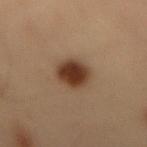Impression: Recorded during total-body skin imaging; not selected for excision or biopsy. Clinical summary: Measured at roughly 4.5 mm in maximum diameter. From the lower back. A lesion tile, about 15 mm wide, cut from a 3D total-body photograph. The lesion-visualizer software estimated an area of roughly 10 mm², an outline eccentricity of about 0.75 (0 = round, 1 = elongated), and two-axis asymmetry of about 0.15. And it measured a mean CIELAB color near L≈30 a*≈16 b*≈24. The software also gave a border-irregularity rating of about 1.5/10, internal color variation of about 4 on a 0–10 scale, and peripheral color asymmetry of about 1. A male patient, about 55 years old. Captured under cross-polarized illumination.